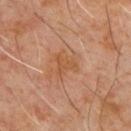| feature | finding |
|---|---|
| workup | no biopsy performed (imaged during a skin exam) |
| patient | male, in their 60s |
| automated metrics | an area of roughly 4.5 mm², a shape eccentricity near 0.55, and a symmetry-axis asymmetry near 0.65; a mean CIELAB color near L≈49 a*≈22 b*≈34, a lesion–skin lightness drop of about 6, and a normalized lesion–skin contrast near 6; a classifier nevus-likeness of about 0/100 and a lesion-detection confidence of about 100/100 |
| lighting | cross-polarized illumination |
| site | the chest |
| acquisition | 15 mm crop, total-body photography |